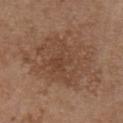– follow-up — no biopsy performed (imaged during a skin exam)
– lighting — white-light
– subject — female, approximately 65 years of age
– automated lesion analysis — internal color variation of about 3.5 on a 0–10 scale and radial color variation of about 1
– image source — total-body-photography crop, ~15 mm field of view
– body site — the chest